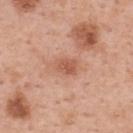| field | value |
|---|---|
| biopsy status | total-body-photography surveillance lesion; no biopsy |
| acquisition | 15 mm crop, total-body photography |
| location | the upper back |
| patient | female, about 50 years old |